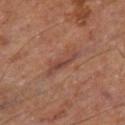| key | value |
|---|---|
| biopsy status | imaged on a skin check; not biopsied |
| subject | male, aged approximately 70 |
| image source | ~15 mm crop, total-body skin-cancer survey |
| size | ≈5 mm |
| location | the right thigh |